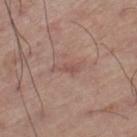The lesion was tiled from a total-body skin photograph and was not biopsied. Imaged with white-light lighting. A close-up tile cropped from a whole-body skin photograph, about 15 mm across. Approximately 4 mm at its widest. An algorithmic analysis of the crop reported a footprint of about 4 mm², a shape eccentricity near 0.9, and a symmetry-axis asymmetry near 0.45. It also reported a mean CIELAB color near L≈52 a*≈21 b*≈23. And it measured an automated nevus-likeness rating near 0 out of 100 and lesion-presence confidence of about 100/100. The lesion is on the leg. A male patient aged approximately 70.